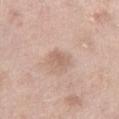  biopsy_status: not biopsied; imaged during a skin examination
  image:
    source: total-body photography crop
    field_of_view_mm: 15
  site: left thigh
  lesion_size:
    long_diameter_mm_approx: 2.5
  patient:
    sex: female
    age_approx: 55